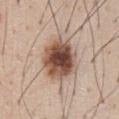A 15 mm crop from a total-body photograph taken for skin-cancer surveillance.
Located on the chest.
A male patient, roughly 60 years of age.
Imaged with white-light lighting.